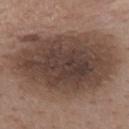Q: Was this lesion biopsied?
A: total-body-photography surveillance lesion; no biopsy
Q: What is the anatomic site?
A: the mid back
Q: What did automated image analysis measure?
A: a footprint of about 85 mm², an outline eccentricity of about 0.75 (0 = round, 1 = elongated), and a shape-asymmetry score of about 0.15 (0 = symmetric); border irregularity of about 2.5 on a 0–10 scale, a within-lesion color-variation index near 6/10, and radial color variation of about 2; a classifier nevus-likeness of about 25/100 and a detector confidence of about 100 out of 100 that the crop contains a lesion
Q: How was this image acquired?
A: 15 mm crop, total-body photography
Q: Who is the patient?
A: female, roughly 45 years of age